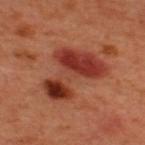| field | value |
|---|---|
| notes | catalogued during a skin exam; not biopsied |
| illumination | cross-polarized |
| subject | male, aged around 50 |
| anatomic site | the back |
| imaging modality | 15 mm crop, total-body photography |